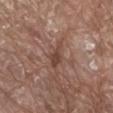The lesion was tiled from a total-body skin photograph and was not biopsied.
From the abdomen.
Imaged with white-light lighting.
The subject is a male approximately 80 years of age.
The recorded lesion diameter is about 3.5 mm.
A lesion tile, about 15 mm wide, cut from a 3D total-body photograph.